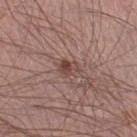No biopsy was performed on this lesion — it was imaged during a full skin examination and was not determined to be concerning.
Cropped from a whole-body photographic skin survey; the tile spans about 15 mm.
A male subject, approximately 55 years of age.
On the right lower leg.